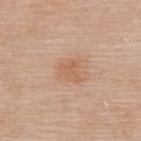Background:
The recorded lesion diameter is about 2.5 mm. Cropped from a whole-body photographic skin survey; the tile spans about 15 mm. The lesion is located on the upper back. The subject is a female aged 53 to 57.